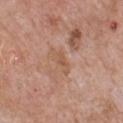<record>
  <biopsy_status>not biopsied; imaged during a skin examination</biopsy_status>
  <patient>
    <sex>male</sex>
    <age_approx>60</age_approx>
  </patient>
  <image>
    <source>total-body photography crop</source>
    <field_of_view_mm>15</field_of_view_mm>
  </image>
  <lesion_size>
    <long_diameter_mm_approx>3.5</long_diameter_mm_approx>
  </lesion_size>
  <site>front of the torso</site>
  <automated_metrics>
    <area_mm2_approx>4.0</area_mm2_approx>
    <eccentricity>0.9</eccentricity>
    <shape_asymmetry>0.35</shape_asymmetry>
    <cielab_L>55</cielab_L>
    <cielab_a>21</cielab_a>
    <cielab_b>31</cielab_b>
    <vs_skin_contrast_norm>5.5</vs_skin_contrast_norm>
    <nevus_likeness_0_100>0</nevus_likeness_0_100>
    <lesion_detection_confidence_0_100>100</lesion_detection_confidence_0_100>
  </automated_metrics>
</record>